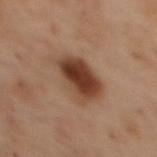biopsy status = imaged on a skin check; not biopsied
site = the upper back
image = 15 mm crop, total-body photography
subject = female, in their mid-50s